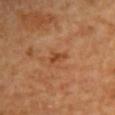* notes · catalogued during a skin exam; not biopsied
* image · ~15 mm tile from a whole-body skin photo
* subject · female
* TBP lesion metrics · an area of roughly 2.5 mm², an eccentricity of roughly 0.85, and two-axis asymmetry of about 0.45
* body site · the upper back